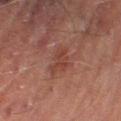The lesion was photographed on a routine skin check and not biopsied; there is no pathology result.
Automated tile analysis of the lesion measured a footprint of about 6 mm², an outline eccentricity of about 0.7 (0 = round, 1 = elongated), and a symmetry-axis asymmetry near 0.35. The software also gave an average lesion color of about L≈33 a*≈20 b*≈23 (CIELAB), about 5 CIELAB-L* units darker than the surrounding skin, and a normalized lesion–skin contrast near 5.5. And it measured a within-lesion color-variation index near 2.5/10 and a peripheral color-asymmetry measure near 1.
Captured under cross-polarized illumination.
The lesion is on the right thigh.
The recorded lesion diameter is about 3.5 mm.
A male subject, aged approximately 70.
A roughly 15 mm field-of-view crop from a total-body skin photograph.